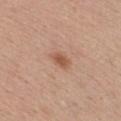The lesion is located on the chest. Imaged with white-light lighting. A 15 mm close-up tile from a total-body photography series done for melanoma screening. The lesion's longest dimension is about 2.5 mm. The patient is a male in their 40s.